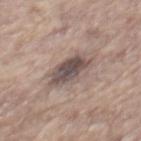follow-up: total-body-photography surveillance lesion; no biopsy | location: the back | acquisition: total-body-photography crop, ~15 mm field of view | automated lesion analysis: roughly 13 lightness units darker than nearby skin; a detector confidence of about 95 out of 100 that the crop contains a lesion | tile lighting: white-light illumination | patient: male, approximately 65 years of age.